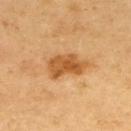Captured during whole-body skin photography for melanoma surveillance; the lesion was not biopsied. A lesion tile, about 15 mm wide, cut from a 3D total-body photograph. Imaged with cross-polarized lighting. The lesion is on the upper back. A female subject roughly 60 years of age.